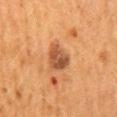workup: total-body-photography surveillance lesion; no biopsy
patient: female, aged approximately 50
acquisition: ~15 mm crop, total-body skin-cancer survey
lesion diameter: ≈3.5 mm
lighting: cross-polarized illumination
anatomic site: the mid back